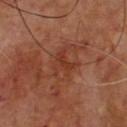Case summary:
- follow-up · imaged on a skin check; not biopsied
- image · total-body-photography crop, ~15 mm field of view
- lesion size · about 3 mm
- TBP lesion metrics · a border-irregularity rating of about 7.5/10, a color-variation rating of about 2/10, and radial color variation of about 0.5; a nevus-likeness score of about 0/100
- subject · male, aged 63–67